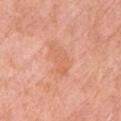workup: catalogued during a skin exam; not biopsied | illumination: white-light | anatomic site: the left upper arm | subject: female, aged 73 to 77 | automated lesion analysis: a footprint of about 6.5 mm² and an outline eccentricity of about 0.9 (0 = round, 1 = elongated); a lesion color around L≈64 a*≈26 b*≈37 in CIELAB and a lesion-to-skin contrast of about 5 (normalized; higher = more distinct) | image source: total-body-photography crop, ~15 mm field of view.